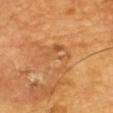Q: Is there a histopathology result?
A: imaged on a skin check; not biopsied
Q: Where on the body is the lesion?
A: the upper back
Q: How was this image acquired?
A: 15 mm crop, total-body photography
Q: What did automated image analysis measure?
A: an automated nevus-likeness rating near 0 out of 100 and a detector confidence of about 90 out of 100 that the crop contains a lesion
Q: Who is the patient?
A: male, in their mid- to late 60s
Q: How large is the lesion?
A: ≈5 mm